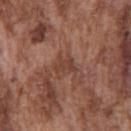Captured during whole-body skin photography for melanoma surveillance; the lesion was not biopsied.
A male subject approximately 75 years of age.
A 15 mm crop from a total-body photograph taken for skin-cancer surveillance.
Captured under white-light illumination.
The recorded lesion diameter is about 3 mm.
The total-body-photography lesion software estimated a shape eccentricity near 0.7 and two-axis asymmetry of about 0.4. And it measured roughly 7 lightness units darker than nearby skin and a normalized border contrast of about 6.
From the chest.